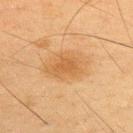| field | value |
|---|---|
| biopsy status | no biopsy performed (imaged during a skin exam) |
| acquisition | ~15 mm tile from a whole-body skin photo |
| diameter | ≈5.5 mm |
| TBP lesion metrics | a border-irregularity index near 1.5/10 and a color-variation rating of about 2.5/10 |
| location | the upper back |
| patient | male, in their mid-30s |
| tile lighting | cross-polarized illumination |